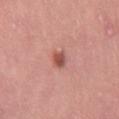Part of a total-body skin-imaging series; this lesion was reviewed on a skin check and was not flagged for biopsy. A 15 mm close-up extracted from a 3D total-body photography capture. Automated image analysis of the tile measured a classifier nevus-likeness of about 95/100 and lesion-presence confidence of about 100/100. A female subject roughly 50 years of age. Measured at roughly 2.5 mm in maximum diameter. The lesion is located on the leg.